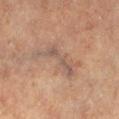subject — female, roughly 60 years of age
anatomic site — the left lower leg
automated metrics — an outline eccentricity of about 0.9 (0 = round, 1 = elongated); roughly 6 lightness units darker than nearby skin; a border-irregularity index near 9/10 and a color-variation rating of about 2.5/10; a nevus-likeness score of about 0/100 and a detector confidence of about 55 out of 100 that the crop contains a lesion
size — ~6.5 mm (longest diameter)
image source — ~15 mm crop, total-body skin-cancer survey